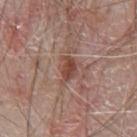follow-up: no biopsy performed (imaged during a skin exam) | acquisition: 15 mm crop, total-body photography | subject: male, in their mid- to late 60s | body site: the chest | illumination: white-light illumination | diameter: ≈3.5 mm | image-analysis metrics: a lesion area of about 6 mm², an eccentricity of roughly 0.7, and two-axis asymmetry of about 0.45; a lesion-to-skin contrast of about 7.5 (normalized; higher = more distinct); a peripheral color-asymmetry measure near 1.5.